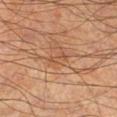biopsy status: imaged on a skin check; not biopsied.